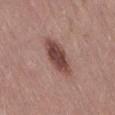The lesion is on the lower back. An algorithmic analysis of the crop reported a lesion area of about 11 mm² and an outline eccentricity of about 0.85 (0 = round, 1 = elongated). It also reported a lesion color around L≈45 a*≈21 b*≈22 in CIELAB and about 14 CIELAB-L* units darker than the surrounding skin. It also reported a peripheral color-asymmetry measure near 1. A female patient aged approximately 40. About 5 mm across. The tile uses white-light illumination. A 15 mm close-up tile from a total-body photography series done for melanoma screening.